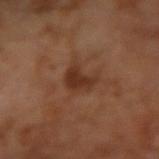{"patient": {"sex": "male", "age_approx": 65}, "lesion_size": {"long_diameter_mm_approx": 3.0}, "automated_metrics": {"area_mm2_approx": 5.5, "eccentricity": 0.75, "shape_asymmetry": 0.45}, "image": {"source": "total-body photography crop", "field_of_view_mm": 15}}